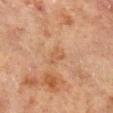Q: Was a biopsy performed?
A: catalogued during a skin exam; not biopsied
Q: What is the imaging modality?
A: ~15 mm tile from a whole-body skin photo
Q: What is the lesion's diameter?
A: ~3 mm (longest diameter)
Q: Illumination type?
A: cross-polarized
Q: Lesion location?
A: the left lower leg
Q: Patient demographics?
A: male, aged 63 to 67